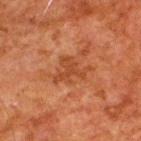Assessment: The lesion was photographed on a routine skin check and not biopsied; there is no pathology result. Image and clinical context: Located on the upper back. Automated tile analysis of the lesion measured an average lesion color of about L≈36 a*≈24 b*≈32 (CIELAB) and a lesion–skin lightness drop of about 6. The analysis additionally found a nevus-likeness score of about 0/100 and lesion-presence confidence of about 100/100. The subject is a male in their 80s. The tile uses cross-polarized illumination. A region of skin cropped from a whole-body photographic capture, roughly 15 mm wide.